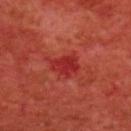biopsy_status: not biopsied; imaged during a skin examination
lighting: cross-polarized
patient:
  sex: male
  age_approx: 60
automated_metrics:
  cielab_L: 33
  cielab_a: 41
  cielab_b: 30
  vs_skin_darker_L: 8.0
  border_irregularity_0_10: 2.5
  color_variation_0_10: 2.0
  lesion_detection_confidence_0_100: 100
image:
  source: total-body photography crop
  field_of_view_mm: 15
site: upper back
lesion_size:
  long_diameter_mm_approx: 3.0